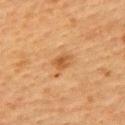notes = catalogued during a skin exam; not biopsied | diameter = ~3.5 mm (longest diameter) | automated metrics = a footprint of about 5.5 mm², a shape eccentricity near 0.75, and a symmetry-axis asymmetry near 0.45; an average lesion color of about L≈46 a*≈20 b*≈35 (CIELAB), a lesion–skin lightness drop of about 7, and a normalized lesion–skin contrast near 6.5; an automated nevus-likeness rating near 60 out of 100 and a lesion-detection confidence of about 100/100 | body site = the upper back | image = total-body-photography crop, ~15 mm field of view | patient = female, approximately 55 years of age | illumination = cross-polarized.